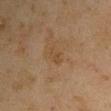Impression:
The lesion was photographed on a routine skin check and not biopsied; there is no pathology result.
Context:
A close-up tile cropped from a whole-body skin photograph, about 15 mm across. A male subject in their mid- to late 40s. Imaged with cross-polarized lighting. On the arm.